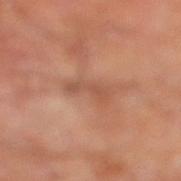The lesion was photographed on a routine skin check and not biopsied; there is no pathology result.